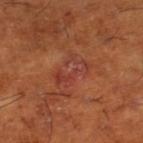Case summary:
– workup — catalogued during a skin exam; not biopsied
– acquisition — total-body-photography crop, ~15 mm field of view
– site — the right lower leg
– subject — male, approximately 65 years of age
– diameter — ≈4 mm